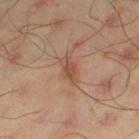patient:
  sex: male
  age_approx: 45
lesion_size:
  long_diameter_mm_approx: 3.0
site: left thigh
image:
  source: total-body photography crop
  field_of_view_mm: 15